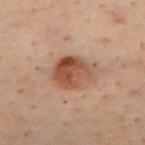Findings:
• biopsy status: imaged on a skin check; not biopsied
• image: 15 mm crop, total-body photography
• anatomic site: the back
• lighting: cross-polarized illumination
• subject: male, aged approximately 50
• lesion size: ~4.5 mm (longest diameter)